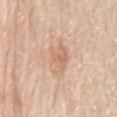{"biopsy_status": "not biopsied; imaged during a skin examination", "image": {"source": "total-body photography crop", "field_of_view_mm": 15}, "lesion_size": {"long_diameter_mm_approx": 4.0}, "patient": {"sex": "male", "age_approx": 80}, "site": "mid back"}